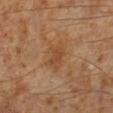biopsy_status: not biopsied; imaged during a skin examination
image:
  source: total-body photography crop
  field_of_view_mm: 15
patient:
  sex: male
  age_approx: 60
lighting: cross-polarized
site: left lower leg